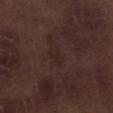This lesion was catalogued during total-body skin photography and was not selected for biopsy.
Longest diameter approximately 3 mm.
A male patient, aged around 70.
On the right lower leg.
The tile uses white-light illumination.
This image is a 15 mm lesion crop taken from a total-body photograph.
Automated image analysis of the tile measured a footprint of about 3 mm², an eccentricity of roughly 0.9, and a shape-asymmetry score of about 0.35 (0 = symmetric). The software also gave a border-irregularity index near 3.5/10, internal color variation of about 0.5 on a 0–10 scale, and a peripheral color-asymmetry measure near 0. And it measured lesion-presence confidence of about 100/100.